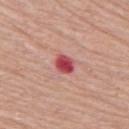Q: Was this lesion biopsied?
A: total-body-photography surveillance lesion; no biopsy
Q: Lesion location?
A: the back
Q: What kind of image is this?
A: 15 mm crop, total-body photography
Q: Who is the patient?
A: male, aged approximately 70
Q: How was the tile lit?
A: white-light